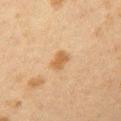Case summary:
• biopsy status — catalogued during a skin exam; not biopsied
• subject — female, in their 40s
• image — ~15 mm tile from a whole-body skin photo
• location — the left upper arm
• diameter — about 2.5 mm